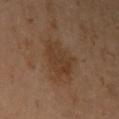notes: total-body-photography surveillance lesion; no biopsy
lesion size: ≈4.5 mm
site: the left upper arm
patient: female, in their mid-40s
image source: ~15 mm crop, total-body skin-cancer survey
illumination: cross-polarized